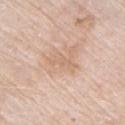* notes: no biopsy performed (imaged during a skin exam)
* automated metrics: a lesion color around L≈66 a*≈18 b*≈32 in CIELAB, a lesion–skin lightness drop of about 7, and a lesion-to-skin contrast of about 5 (normalized; higher = more distinct)
* image: ~15 mm tile from a whole-body skin photo
* anatomic site: the chest
* size: ≈3.5 mm
* patient: male, roughly 75 years of age